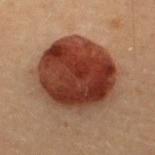Recorded during total-body skin imaging; not selected for excision or biopsy.
About 8.5 mm across.
The subject is a female about 30 years old.
This is a cross-polarized tile.
Cropped from a whole-body photographic skin survey; the tile spans about 15 mm.
The lesion is on the upper back.